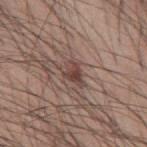Findings:
– illumination: white-light illumination
– acquisition: total-body-photography crop, ~15 mm field of view
– TBP lesion metrics: a footprint of about 4.5 mm², a shape eccentricity near 0.75, and a shape-asymmetry score of about 0.45 (0 = symmetric); about 10 CIELAB-L* units darker than the surrounding skin; a nevus-likeness score of about 40/100 and lesion-presence confidence of about 100/100
– site: the mid back
– diameter: about 3 mm
– subject: male, roughly 60 years of age